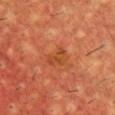workup: total-body-photography surveillance lesion; no biopsy
acquisition: total-body-photography crop, ~15 mm field of view
diameter: about 2.5 mm
tile lighting: cross-polarized illumination
subject: male, roughly 55 years of age
anatomic site: the upper back
automated lesion analysis: a mean CIELAB color near L≈40 a*≈27 b*≈37 and a normalized border contrast of about 5.5; a nevus-likeness score of about 0/100 and a detector confidence of about 100 out of 100 that the crop contains a lesion